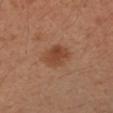Case summary:
• follow-up · catalogued during a skin exam; not biopsied
• lighting · cross-polarized illumination
• acquisition · 15 mm crop, total-body photography
• subject · female, approximately 40 years of age
• body site · the left forearm